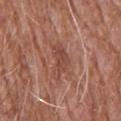| key | value |
|---|---|
| follow-up | catalogued during a skin exam; not biopsied |
| tile lighting | white-light |
| patient | male, approximately 60 years of age |
| acquisition | total-body-photography crop, ~15 mm field of view |
| lesion size | ≈4.5 mm |
| body site | the chest |